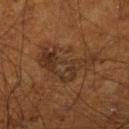follow-up = catalogued during a skin exam; not biopsied | subject = male, aged 58 to 62 | lesion size = about 7.5 mm | anatomic site = the left lower leg | acquisition = total-body-photography crop, ~15 mm field of view.